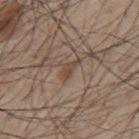<case>
<biopsy_status>not biopsied; imaged during a skin examination</biopsy_status>
<patient>
  <sex>male</sex>
  <age_approx>45</age_approx>
</patient>
<site>mid back</site>
<automated_metrics>
  <nevus_likeness_0_100>70</nevus_likeness_0_100>
  <lesion_detection_confidence_0_100>70</lesion_detection_confidence_0_100>
</automated_metrics>
<lighting>white-light</lighting>
<image>
  <source>total-body photography crop</source>
  <field_of_view_mm>15</field_of_view_mm>
</image>
<lesion_size>
  <long_diameter_mm_approx>3.0</long_diameter_mm_approx>
</lesion_size>
</case>